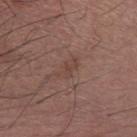Clinical impression:
Recorded during total-body skin imaging; not selected for excision or biopsy.
Acquisition and patient details:
A lesion tile, about 15 mm wide, cut from a 3D total-body photograph. On the left forearm. The patient is a male aged around 65.